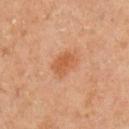This lesion was catalogued during total-body skin photography and was not selected for biopsy. A male patient about 60 years old. Cropped from a whole-body photographic skin survey; the tile spans about 15 mm. Automated image analysis of the tile measured a lesion color around L≈53 a*≈25 b*≈36 in CIELAB and about 8 CIELAB-L* units darker than the surrounding skin. It also reported a classifier nevus-likeness of about 80/100 and a lesion-detection confidence of about 100/100. Captured under cross-polarized illumination. Approximately 3 mm at its widest. From the left upper arm.